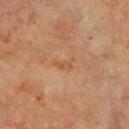Clinical impression: Recorded during total-body skin imaging; not selected for excision or biopsy. Image and clinical context: A region of skin cropped from a whole-body photographic capture, roughly 15 mm wide. A male subject, about 65 years old. Located on the chest. Captured under cross-polarized illumination. About 3 mm across.